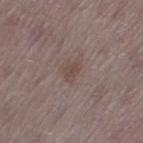<record>
  <biopsy_status>not biopsied; imaged during a skin examination</biopsy_status>
  <patient>
    <sex>female</sex>
    <age_approx>50</age_approx>
  </patient>
  <lighting>white-light</lighting>
  <image>
    <source>total-body photography crop</source>
    <field_of_view_mm>15</field_of_view_mm>
  </image>
  <lesion_size>
    <long_diameter_mm_approx>2.5</long_diameter_mm_approx>
  </lesion_size>
  <site>left thigh</site>
</record>